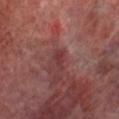Q: Was this lesion biopsied?
A: catalogued during a skin exam; not biopsied
Q: Patient demographics?
A: male, aged approximately 70
Q: How was this image acquired?
A: ~15 mm crop, total-body skin-cancer survey
Q: Lesion location?
A: the right lower leg